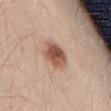Assessment:
No biopsy was performed on this lesion — it was imaged during a full skin examination and was not determined to be concerning.
Background:
On the right thigh. The subject is a male aged 43–47. A close-up tile cropped from a whole-body skin photograph, about 15 mm across. The tile uses white-light illumination. Approximately 3.5 mm at its widest.